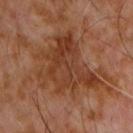Impression:
No biopsy was performed on this lesion — it was imaged during a full skin examination and was not determined to be concerning.
Context:
This is a cross-polarized tile. The patient is a male aged around 60. A roughly 15 mm field-of-view crop from a total-body skin photograph. The recorded lesion diameter is about 8 mm. The lesion is on the front of the torso.